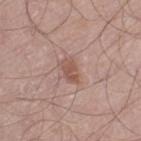| key | value |
|---|---|
| follow-up | catalogued during a skin exam; not biopsied |
| image source | total-body-photography crop, ~15 mm field of view |
| patient | male, in their 70s |
| tile lighting | white-light illumination |
| location | the leg |
| diameter | about 3 mm |
| TBP lesion metrics | a lesion-detection confidence of about 100/100 |